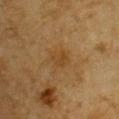Notes:
– notes: catalogued during a skin exam; not biopsied
– lesion size: about 2.5 mm
– subject: male, aged 83 or over
– imaging modality: ~15 mm crop, total-body skin-cancer survey
– site: the front of the torso
– illumination: cross-polarized